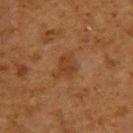A close-up tile cropped from a whole-body skin photograph, about 15 mm across. Measured at roughly 3.5 mm in maximum diameter. A male subject approximately 60 years of age. The total-body-photography lesion software estimated a shape eccentricity near 0.7 and two-axis asymmetry of about 0.4. And it measured a mean CIELAB color near L≈33 a*≈19 b*≈30, a lesion–skin lightness drop of about 6, and a normalized border contrast of about 6. Located on the upper back. Captured under cross-polarized illumination.